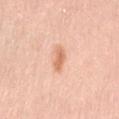lesion diameter: ≈3 mm | automated lesion analysis: an area of roughly 3.5 mm², an eccentricity of roughly 0.9, and a shape-asymmetry score of about 0.3 (0 = symmetric); a nevus-likeness score of about 80/100 and a lesion-detection confidence of about 100/100 | image: 15 mm crop, total-body photography | illumination: white-light illumination | location: the abdomen | patient: female, aged 38 to 42.